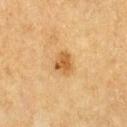Part of a total-body skin-imaging series; this lesion was reviewed on a skin check and was not flagged for biopsy.
A 15 mm crop from a total-body photograph taken for skin-cancer surveillance.
A female subject aged around 55.
About 2.5 mm across.
From the left thigh.
Captured under cross-polarized illumination.